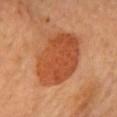Recorded during total-body skin imaging; not selected for excision or biopsy. A 15 mm close-up tile from a total-body photography series done for melanoma screening. A female patient, aged around 60. Located on the chest.